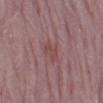| key | value |
|---|---|
| biopsy status | imaged on a skin check; not biopsied |
| site | the left lower leg |
| imaging modality | 15 mm crop, total-body photography |
| image-analysis metrics | about 6 CIELAB-L* units darker than the surrounding skin and a lesion-to-skin contrast of about 5.5 (normalized; higher = more distinct); a lesion-detection confidence of about 95/100 |
| subject | female, aged approximately 65 |